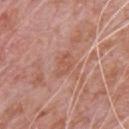This lesion was catalogued during total-body skin photography and was not selected for biopsy.
Located on the chest.
Measured at roughly 3.5 mm in maximum diameter.
Imaged with white-light lighting.
The patient is a male aged approximately 80.
A close-up tile cropped from a whole-body skin photograph, about 15 mm across.